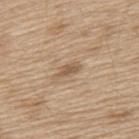biopsy_status: not biopsied; imaged during a skin examination
lesion_size:
  long_diameter_mm_approx: 3.0
patient:
  sex: male
  age_approx: 70
automated_metrics:
  area_mm2_approx: 3.0
  shape_asymmetry: 0.3
  cielab_L: 55
  cielab_a: 16
  cielab_b: 32
  vs_skin_contrast_norm: 7.0
  nevus_likeness_0_100: 0
  lesion_detection_confidence_0_100: 95
image:
  source: total-body photography crop
  field_of_view_mm: 15
site: upper back